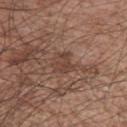Recorded during total-body skin imaging; not selected for excision or biopsy. From the left upper arm. A male patient, about 65 years old. The recorded lesion diameter is about 3 mm. The lesion-visualizer software estimated a shape-asymmetry score of about 0.35 (0 = symmetric). The software also gave an average lesion color of about L≈42 a*≈18 b*≈25 (CIELAB), a lesion–skin lightness drop of about 7, and a normalized border contrast of about 6. It also reported a classifier nevus-likeness of about 0/100 and a lesion-detection confidence of about 90/100. Cropped from a total-body skin-imaging series; the visible field is about 15 mm.